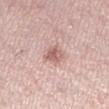Part of a total-body skin-imaging series; this lesion was reviewed on a skin check and was not flagged for biopsy. Measured at roughly 2.5 mm in maximum diameter. A 15 mm crop from a total-body photograph taken for skin-cancer surveillance. Located on the leg. The lesion-visualizer software estimated a footprint of about 4.5 mm², an outline eccentricity of about 0.65 (0 = round, 1 = elongated), and a shape-asymmetry score of about 0.25 (0 = symmetric). And it measured a nevus-likeness score of about 15/100 and a detector confidence of about 100 out of 100 that the crop contains a lesion. A female patient in their 50s.